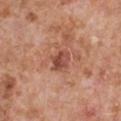This lesion was catalogued during total-body skin photography and was not selected for biopsy. This is a white-light tile. The lesion is on the chest. Cropped from a whole-body photographic skin survey; the tile spans about 15 mm. Automated image analysis of the tile measured a footprint of about 5.5 mm², a shape eccentricity near 0.55, and two-axis asymmetry of about 0.3. It also reported a mean CIELAB color near L≈49 a*≈26 b*≈30, about 11 CIELAB-L* units darker than the surrounding skin, and a lesion-to-skin contrast of about 7.5 (normalized; higher = more distinct). And it measured a border-irregularity index near 2.5/10, internal color variation of about 4.5 on a 0–10 scale, and a peripheral color-asymmetry measure near 2. Measured at roughly 3 mm in maximum diameter. A male subject, in their mid- to late 60s.